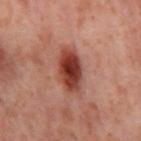follow-up=imaged on a skin check; not biopsied
lesion size=~5 mm (longest diameter)
body site=the right thigh
image source=total-body-photography crop, ~15 mm field of view
patient=female, aged around 55